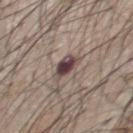| field | value |
|---|---|
| workup | imaged on a skin check; not biopsied |
| anatomic site | the chest |
| subject | male, aged 43 to 47 |
| image | ~15 mm crop, total-body skin-cancer survey |
| lesion size | ≈3.5 mm |